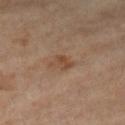The lesion was photographed on a routine skin check and not biopsied; there is no pathology result.
The tile uses cross-polarized illumination.
Automated image analysis of the tile measured an area of roughly 4 mm², a shape eccentricity near 0.7, and a symmetry-axis asymmetry near 0.35. And it measured a border-irregularity index near 3.5/10, a within-lesion color-variation index near 2.5/10, and a peripheral color-asymmetry measure near 1. The analysis additionally found an automated nevus-likeness rating near 0 out of 100.
This image is a 15 mm lesion crop taken from a total-body photograph.
The lesion's longest dimension is about 2.5 mm.
From the right lower leg.
A female subject aged 73–77.